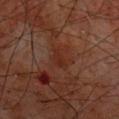{
  "biopsy_status": "not biopsied; imaged during a skin examination",
  "image": {
    "source": "total-body photography crop",
    "field_of_view_mm": 15
  },
  "automated_metrics": {
    "vs_skin_darker_L": 5.0,
    "vs_skin_contrast_norm": 5.5,
    "border_irregularity_0_10": 4.0,
    "color_variation_0_10": 1.5,
    "nevus_likeness_0_100": 0
  },
  "site": "upper back",
  "lesion_size": {
    "long_diameter_mm_approx": 3.0
  },
  "lighting": "cross-polarized",
  "patient": {
    "sex": "male",
    "age_approx": 60
  }
}